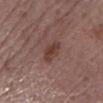Captured during whole-body skin photography for melanoma surveillance; the lesion was not biopsied.
Automated tile analysis of the lesion measured a peripheral color-asymmetry measure near 0.5. The analysis additionally found lesion-presence confidence of about 100/100.
A 15 mm crop from a total-body photograph taken for skin-cancer surveillance.
A female subject aged 63 to 67.
The lesion is on the left lower leg.